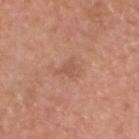Cropped from a whole-body photographic skin survey; the tile spans about 15 mm. On the head or neck. A male patient aged 53 to 57.